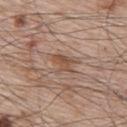follow-up: catalogued during a skin exam; not biopsied
site: the upper back
automated lesion analysis: a footprint of about 4.5 mm², an eccentricity of roughly 0.8, and a symmetry-axis asymmetry near 0.4; about 8 CIELAB-L* units darker than the surrounding skin and a lesion-to-skin contrast of about 6.5 (normalized; higher = more distinct); border irregularity of about 5 on a 0–10 scale, a within-lesion color-variation index near 3/10, and peripheral color asymmetry of about 1
illumination: white-light
image source: ~15 mm crop, total-body skin-cancer survey
lesion diameter: about 3.5 mm
patient: male, aged 63–67